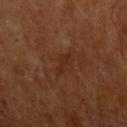Impression:
Captured during whole-body skin photography for melanoma surveillance; the lesion was not biopsied.
Background:
On the left upper arm. The patient is a male roughly 65 years of age. A roughly 15 mm field-of-view crop from a total-body skin photograph. The total-body-photography lesion software estimated an outline eccentricity of about 0.9 (0 = round, 1 = elongated) and a shape-asymmetry score of about 0.3 (0 = symmetric). And it measured internal color variation of about 0.5 on a 0–10 scale and peripheral color asymmetry of about 0. Captured under cross-polarized illumination.